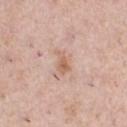Findings:
• notes — no biopsy performed (imaged during a skin exam)
• location — the chest
• automated lesion analysis — a lesion area of about 4 mm², a shape eccentricity near 0.85, and a symmetry-axis asymmetry near 0.35; an automated nevus-likeness rating near 20 out of 100 and lesion-presence confidence of about 100/100
• image — 15 mm crop, total-body photography
• illumination — white-light
• lesion size — ≈3 mm
• subject — male, approximately 40 years of age